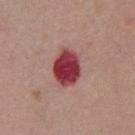{
  "biopsy_status": "not biopsied; imaged during a skin examination",
  "automated_metrics": {
    "area_mm2_approx": 12.0,
    "eccentricity": 0.75,
    "shape_asymmetry": 0.15,
    "vs_skin_darker_L": 19.0,
    "vs_skin_contrast_norm": 13.5,
    "color_variation_0_10": 4.5,
    "nevus_likeness_0_100": 0,
    "lesion_detection_confidence_0_100": 100
  },
  "image": {
    "source": "total-body photography crop",
    "field_of_view_mm": 15
  },
  "site": "abdomen",
  "patient": {
    "sex": "male",
    "age_approx": 55
  }
}